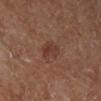biopsy status: imaged on a skin check; not biopsied, image-analysis metrics: a lesion color around L≈37 a*≈21 b*≈25 in CIELAB and a normalized border contrast of about 6.5; an automated nevus-likeness rating near 10 out of 100, size: ≈3 mm, lighting: cross-polarized illumination, location: the right lower leg, subject: female, image: ~15 mm tile from a whole-body skin photo.